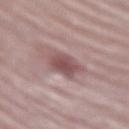Imaged during a routine full-body skin examination; the lesion was not biopsied and no histopathology is available. The lesion is located on the right upper arm. A close-up tile cropped from a whole-body skin photograph, about 15 mm across. About 3.5 mm across. Imaged with white-light lighting. A female subject aged around 65.